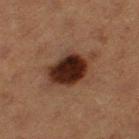Part of a total-body skin-imaging series; this lesion was reviewed on a skin check and was not flagged for biopsy. Cropped from a total-body skin-imaging series; the visible field is about 15 mm. Longest diameter approximately 6 mm. Located on the left thigh. The tile uses cross-polarized illumination. A female subject, aged 38 to 42.